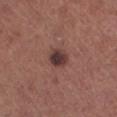{"patient": {"sex": "female", "age_approx": 65}, "image": {"source": "total-body photography crop", "field_of_view_mm": 15}, "lighting": "white-light", "site": "leg"}